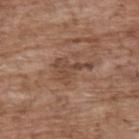No biopsy was performed on this lesion — it was imaged during a full skin examination and was not determined to be concerning. A lesion tile, about 15 mm wide, cut from a 3D total-body photograph. On the back. A male patient, in their 70s.